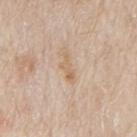Part of a total-body skin-imaging series; this lesion was reviewed on a skin check and was not flagged for biopsy. A roughly 15 mm field-of-view crop from a total-body skin photograph. On the mid back. A male patient roughly 65 years of age. Imaged with white-light lighting. Longest diameter approximately 3.5 mm.